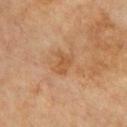Imaged during a routine full-body skin examination; the lesion was not biopsied and no histopathology is available. The recorded lesion diameter is about 3 mm. Located on the chest. This image is a 15 mm lesion crop taken from a total-body photograph. A male subject, aged approximately 60.